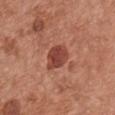Part of a total-body skin-imaging series; this lesion was reviewed on a skin check and was not flagged for biopsy. A lesion tile, about 15 mm wide, cut from a 3D total-body photograph. Measured at roughly 4 mm in maximum diameter. Imaged with white-light lighting. The lesion is located on the chest. A female patient, aged approximately 65.